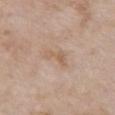Impression:
This lesion was catalogued during total-body skin photography and was not selected for biopsy.
Image and clinical context:
A 15 mm crop from a total-body photograph taken for skin-cancer surveillance. The lesion-visualizer software estimated an area of roughly 4 mm², an outline eccentricity of about 0.9 (0 = round, 1 = elongated), and a symmetry-axis asymmetry near 0.55. It also reported a mean CIELAB color near L≈60 a*≈16 b*≈30, roughly 7 lightness units darker than nearby skin, and a normalized lesion–skin contrast near 5.5. The software also gave a border-irregularity index near 5.5/10 and a within-lesion color-variation index near 0.5/10. A female subject in their mid-70s. The lesion's longest dimension is about 3.5 mm. Imaged with white-light lighting. From the chest.